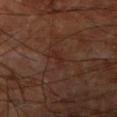biopsy status: imaged on a skin check; not biopsied | diameter: ≈2.5 mm | anatomic site: the right upper arm | subject: about 65 years old | imaging modality: ~15 mm crop, total-body skin-cancer survey | lighting: cross-polarized | image-analysis metrics: a border-irregularity index near 3.5/10 and a peripheral color-asymmetry measure near 0.